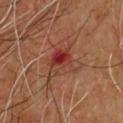follow-up=no biopsy performed (imaged during a skin exam) | location=the chest | patient=male, approximately 65 years of age | lesion diameter=≈6 mm | image-analysis metrics=a lesion–skin lightness drop of about 7; a nevus-likeness score of about 0/100 and lesion-presence confidence of about 100/100 | lighting=cross-polarized | image=15 mm crop, total-body photography.